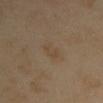A lesion tile, about 15 mm wide, cut from a 3D total-body photograph.
On the chest.
The subject is a female aged 33 to 37.